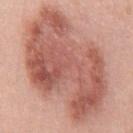The lesion was tiled from a total-body skin photograph and was not biopsied.
An algorithmic analysis of the crop reported a lesion color around L≈57 a*≈25 b*≈27 in CIELAB and a normalized border contrast of about 8.5. And it measured a border-irregularity index near 3.5/10 and a color-variation rating of about 7/10. And it measured a lesion-detection confidence of about 100/100.
Captured under white-light illumination.
A roughly 15 mm field-of-view crop from a total-body skin photograph.
The patient is a female aged around 40.
Located on the chest.
Approximately 14 mm at its widest.